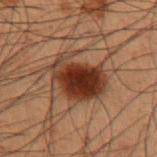Recorded during total-body skin imaging; not selected for excision or biopsy. The lesion is located on the left upper arm. A lesion tile, about 15 mm wide, cut from a 3D total-body photograph. Longest diameter approximately 5.5 mm. A male subject, about 55 years old. Imaged with cross-polarized lighting.